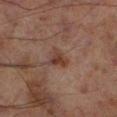Clinical summary:
This image is a 15 mm lesion crop taken from a total-body photograph. The lesion is on the leg. About 2.5 mm across. A male subject aged 68–72.